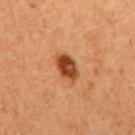biopsy status: no biopsy performed (imaged during a skin exam)